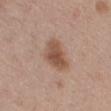The subject is a female roughly 40 years of age. The recorded lesion diameter is about 4.5 mm. The tile uses white-light illumination. A 15 mm crop from a total-body photograph taken for skin-cancer surveillance. The lesion is located on the mid back.